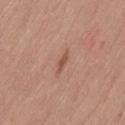Impression:
This lesion was catalogued during total-body skin photography and was not selected for biopsy.
Context:
The lesion is located on the left thigh. A 15 mm close-up tile from a total-body photography series done for melanoma screening. The recorded lesion diameter is about 2.5 mm. The subject is a female approximately 45 years of age. The tile uses white-light illumination.